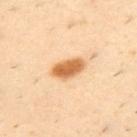Case summary:
• workup: imaged on a skin check; not biopsied
• acquisition: 15 mm crop, total-body photography
• size: ≈4 mm
• anatomic site: the back
• lighting: cross-polarized
• patient: male, aged around 40